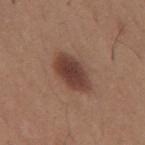biopsy status: imaged on a skin check; not biopsied | diameter: ≈5 mm | automated metrics: a footprint of about 11 mm², a shape eccentricity near 0.85, and a symmetry-axis asymmetry near 0.15; a classifier nevus-likeness of about 95/100 and a detector confidence of about 100 out of 100 that the crop contains a lesion | subject: male, approximately 65 years of age | imaging modality: total-body-photography crop, ~15 mm field of view | illumination: white-light | body site: the mid back.